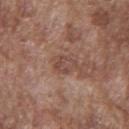<case>
<biopsy_status>not biopsied; imaged during a skin examination</biopsy_status>
<lighting>white-light</lighting>
<site>chest</site>
<lesion_size>
  <long_diameter_mm_approx>2.5</long_diameter_mm_approx>
</lesion_size>
<image>
  <source>total-body photography crop</source>
  <field_of_view_mm>15</field_of_view_mm>
</image>
<automated_metrics>
  <area_mm2_approx>4.5</area_mm2_approx>
  <shape_asymmetry>0.3</shape_asymmetry>
  <vs_skin_contrast_norm>5.5</vs_skin_contrast_norm>
  <nevus_likeness_0_100>0</nevus_likeness_0_100>
  <lesion_detection_confidence_0_100>100</lesion_detection_confidence_0_100>
</automated_metrics>
<patient>
  <sex>male</sex>
  <age_approx>75</age_approx>
</patient>
</case>